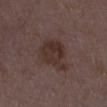Impression:
No biopsy was performed on this lesion — it was imaged during a full skin examination and was not determined to be concerning.
Clinical summary:
A close-up tile cropped from a whole-body skin photograph, about 15 mm across. From the left lower leg. A female subject about 35 years old. Automated image analysis of the tile measured an area of roughly 13 mm², a shape eccentricity near 0.75, and a symmetry-axis asymmetry near 0.3. It also reported a lesion color around L≈31 a*≈16 b*≈19 in CIELAB. The software also gave a classifier nevus-likeness of about 10/100 and lesion-presence confidence of about 100/100.